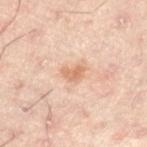Impression: Imaged during a routine full-body skin examination; the lesion was not biopsied and no histopathology is available. Image and clinical context: Cropped from a whole-body photographic skin survey; the tile spans about 15 mm. The lesion is on the left lower leg. A male subject, aged 48–52. Approximately 2.5 mm at its widest.